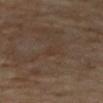{
  "biopsy_status": "not biopsied; imaged during a skin examination",
  "patient": {
    "sex": "female",
    "age_approx": 45
  },
  "lighting": "cross-polarized",
  "automated_metrics": {
    "cielab_L": 35,
    "cielab_a": 14,
    "cielab_b": 25,
    "vs_skin_darker_L": 3.0,
    "border_irregularity_0_10": 3.0,
    "color_variation_0_10": 0.0
  },
  "image": {
    "source": "total-body photography crop",
    "field_of_view_mm": 15
  },
  "lesion_size": {
    "long_diameter_mm_approx": 1.5
  },
  "site": "left forearm"
}